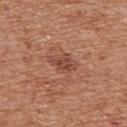Notes:
* follow-up · imaged on a skin check; not biopsied
* patient · male, about 65 years old
* imaging modality · 15 mm crop, total-body photography
* site · the upper back